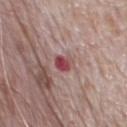biopsy status: catalogued during a skin exam; not biopsied | lesion diameter: about 2.5 mm | location: the chest | acquisition: total-body-photography crop, ~15 mm field of view | subject: male, in their 70s.